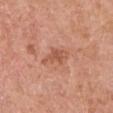Assessment: Imaged during a routine full-body skin examination; the lesion was not biopsied and no histopathology is available. Image and clinical context: The lesion is located on the right upper arm. A close-up tile cropped from a whole-body skin photograph, about 15 mm across. A male subject roughly 70 years of age.